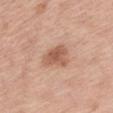Clinical impression:
This lesion was catalogued during total-body skin photography and was not selected for biopsy.
Background:
Approximately 4 mm at its widest. A female subject aged approximately 40. Cropped from a total-body skin-imaging series; the visible field is about 15 mm. Imaged with white-light lighting. The lesion is on the left forearm.